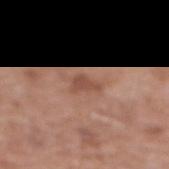On the mid back.
About 2.5 mm across.
This is a white-light tile.
A female subject, approximately 75 years of age.
A 15 mm close-up extracted from a 3D total-body photography capture.
Automated tile analysis of the lesion measured a lesion area of about 3 mm², an eccentricity of roughly 0.8, and a symmetry-axis asymmetry near 0.5. And it measured a lesion color around L≈49 a*≈22 b*≈28 in CIELAB, a lesion–skin lightness drop of about 9, and a lesion-to-skin contrast of about 6.5 (normalized; higher = more distinct).